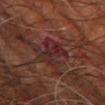| field | value |
|---|---|
| biopsy status | catalogued during a skin exam; not biopsied |
| location | the right leg |
| patient | male, aged approximately 60 |
| image-analysis metrics | a lesion color around L≈25 a*≈22 b*≈19 in CIELAB, a lesion–skin lightness drop of about 6, and a normalized lesion–skin contrast near 7.5 |
| acquisition | 15 mm crop, total-body photography |